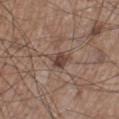Image and clinical context:
Automated tile analysis of the lesion measured a footprint of about 5 mm², an eccentricity of roughly 0.7, and a symmetry-axis asymmetry near 0.35. This is a white-light tile. The subject is a male aged 63–67. Located on the leg. A lesion tile, about 15 mm wide, cut from a 3D total-body photograph. Measured at roughly 3 mm in maximum diameter.